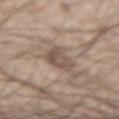Assessment:
The lesion was tiled from a total-body skin photograph and was not biopsied.
Clinical summary:
The lesion-visualizer software estimated a lesion color around L≈51 a*≈15 b*≈23 in CIELAB, a lesion–skin lightness drop of about 10, and a normalized lesion–skin contrast near 7. The analysis additionally found a border-irregularity index near 3/10 and a color-variation rating of about 3.5/10. From the left forearm. This is a white-light tile. A 15 mm close-up tile from a total-body photography series done for melanoma screening. A male subject aged around 30.